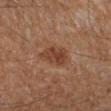This lesion was catalogued during total-body skin photography and was not selected for biopsy.
Captured under cross-polarized illumination.
Located on the right forearm.
A male subject aged approximately 65.
A 15 mm close-up tile from a total-body photography series done for melanoma screening.
The recorded lesion diameter is about 3 mm.
Automated image analysis of the tile measured a shape eccentricity near 0.45 and a shape-asymmetry score of about 0.25 (0 = symmetric). It also reported an average lesion color of about L≈41 a*≈22 b*≈30 (CIELAB) and a normalized lesion–skin contrast near 7.5. It also reported a border-irregularity rating of about 3/10, a within-lesion color-variation index near 3.5/10, and peripheral color asymmetry of about 1. The software also gave a nevus-likeness score of about 45/100 and a detector confidence of about 100 out of 100 that the crop contains a lesion.